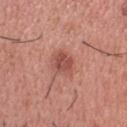The lesion was tiled from a total-body skin photograph and was not biopsied. The subject is a male aged around 35. A roughly 15 mm field-of-view crop from a total-body skin photograph. The lesion is on the head or neck.